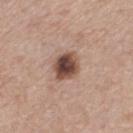Captured during whole-body skin photography for melanoma surveillance; the lesion was not biopsied.
A male subject approximately 55 years of age.
Cropped from a whole-body photographic skin survey; the tile spans about 15 mm.